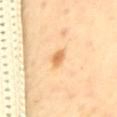The lesion was tiled from a total-body skin photograph and was not biopsied.
From the mid back.
A 15 mm close-up tile from a total-body photography series done for melanoma screening.
The patient is a female approximately 60 years of age.